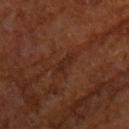Case summary:
- notes · no biopsy performed (imaged during a skin exam)
- subject · male, aged 63–67
- illumination · cross-polarized illumination
- body site · the upper back
- acquisition · total-body-photography crop, ~15 mm field of view
- image-analysis metrics · a mean CIELAB color near L≈24 a*≈20 b*≈25
- lesion diameter · about 3.5 mm